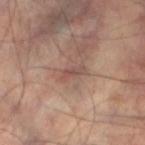Assessment: The lesion was photographed on a routine skin check and not biopsied; there is no pathology result. Acquisition and patient details: The subject is a male aged 48–52. The lesion is on the leg. A region of skin cropped from a whole-body photographic capture, roughly 15 mm wide. This is a cross-polarized tile.